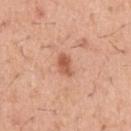Impression:
The lesion was photographed on a routine skin check and not biopsied; there is no pathology result.
Image and clinical context:
A 15 mm crop from a total-body photograph taken for skin-cancer surveillance. The lesion is on the arm. The patient is a male approximately 40 years of age. Imaged with white-light lighting. An algorithmic analysis of the crop reported a footprint of about 4 mm² and a symmetry-axis asymmetry near 0.35. The analysis additionally found a lesion color around L≈58 a*≈26 b*≈34 in CIELAB, about 12 CIELAB-L* units darker than the surrounding skin, and a lesion-to-skin contrast of about 7.5 (normalized; higher = more distinct). It also reported a classifier nevus-likeness of about 80/100 and a detector confidence of about 100 out of 100 that the crop contains a lesion.